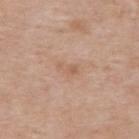Case summary:
– follow-up — total-body-photography surveillance lesion; no biopsy
– site — the upper back
– acquisition — total-body-photography crop, ~15 mm field of view
– diameter — about 2.5 mm
– subject — male, approximately 55 years of age